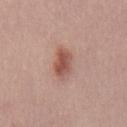Clinical impression: Recorded during total-body skin imaging; not selected for excision or biopsy.